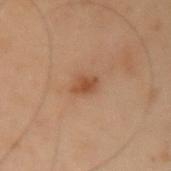Clinical summary:
On the left upper arm. A 15 mm close-up tile from a total-body photography series done for melanoma screening. The tile uses cross-polarized illumination. Measured at roughly 2.5 mm in maximum diameter. A male subject, aged 53–57.